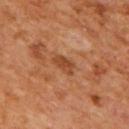{
  "lighting": "cross-polarized",
  "image": {
    "source": "total-body photography crop",
    "field_of_view_mm": 15
  },
  "patient": {
    "sex": "male",
    "age_approx": 65
  },
  "automated_metrics": {
    "area_mm2_approx": 4.0,
    "eccentricity": 0.85,
    "shape_asymmetry": 0.35,
    "cielab_L": 43,
    "cielab_a": 25,
    "cielab_b": 35,
    "vs_skin_darker_L": 9.0,
    "vs_skin_contrast_norm": 7.5,
    "lesion_detection_confidence_0_100": 100
  },
  "site": "back",
  "lesion_size": {
    "long_diameter_mm_approx": 3.0
  }
}